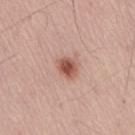This lesion was catalogued during total-body skin photography and was not selected for biopsy. The total-body-photography lesion software estimated an outline eccentricity of about 0.5 (0 = round, 1 = elongated) and two-axis asymmetry of about 0.2. It also reported an average lesion color of about L≈54 a*≈24 b*≈28 (CIELAB) and a normalized border contrast of about 9. Captured under white-light illumination. The subject is a male about 55 years old. This image is a 15 mm lesion crop taken from a total-body photograph. Located on the lower back.